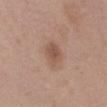The lesion was tiled from a total-body skin photograph and was not biopsied. The lesion is on the abdomen. The total-body-photography lesion software estimated an average lesion color of about L≈53 a*≈19 b*≈28 (CIELAB), about 9 CIELAB-L* units darker than the surrounding skin, and a normalized lesion–skin contrast near 6.5. The subject is a female roughly 65 years of age. The tile uses white-light illumination. A lesion tile, about 15 mm wide, cut from a 3D total-body photograph.